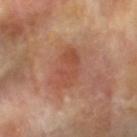Q: Was a biopsy performed?
A: imaged on a skin check; not biopsied
Q: How was the tile lit?
A: cross-polarized illumination
Q: What are the patient's age and sex?
A: female, about 75 years old
Q: What is the imaging modality?
A: ~15 mm crop, total-body skin-cancer survey
Q: What is the lesion's diameter?
A: ≈5 mm
Q: What is the anatomic site?
A: the right forearm